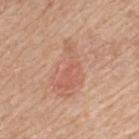Findings:
• biopsy status · imaged on a skin check; not biopsied
• patient · female, roughly 55 years of age
• image source · 15 mm crop, total-body photography
• site · the upper back